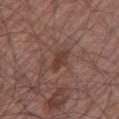The lesion was tiled from a total-body skin photograph and was not biopsied.
The lesion is located on the left upper arm.
The lesion-visualizer software estimated border irregularity of about 3 on a 0–10 scale and peripheral color asymmetry of about 1.
The recorded lesion diameter is about 2.5 mm.
The subject is a male aged 63–67.
A lesion tile, about 15 mm wide, cut from a 3D total-body photograph.
This is a white-light tile.